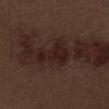Part of a total-body skin-imaging series; this lesion was reviewed on a skin check and was not flagged for biopsy. Captured under white-light illumination. On the front of the torso. Approximately 4.5 mm at its widest. Automated image analysis of the tile measured an average lesion color of about L≈20 a*≈17 b*≈18 (CIELAB) and roughly 7 lightness units darker than nearby skin. The software also gave a border-irregularity rating of about 6/10 and a within-lesion color-variation index near 2.5/10. A region of skin cropped from a whole-body photographic capture, roughly 15 mm wide. The patient is a male in their 70s.